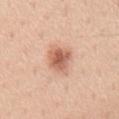– follow-up — total-body-photography surveillance lesion; no biopsy
– lighting — white-light illumination
– size — about 3.5 mm
– image — ~15 mm tile from a whole-body skin photo
– patient — male, roughly 35 years of age
– TBP lesion metrics — an automated nevus-likeness rating near 90 out of 100 and lesion-presence confidence of about 100/100
– site — the back